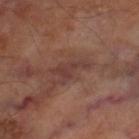workup=no biopsy performed (imaged during a skin exam).